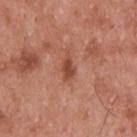Clinical impression:
Captured during whole-body skin photography for melanoma surveillance; the lesion was not biopsied.
Clinical summary:
Captured under white-light illumination. The recorded lesion diameter is about 2.5 mm. From the upper back. A male subject, aged around 55. A 15 mm close-up tile from a total-body photography series done for melanoma screening.